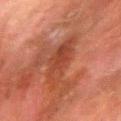| key | value |
|---|---|
| biopsy status | total-body-photography surveillance lesion; no biopsy |
| automated metrics | a footprint of about 13 mm², a shape eccentricity near 0.85, and a symmetry-axis asymmetry near 0.25; a border-irregularity index near 3.5/10 and a peripheral color-asymmetry measure near 1.5 |
| subject | male, approximately 80 years of age |
| anatomic site | the right forearm |
| lesion diameter | about 5.5 mm |
| lighting | cross-polarized illumination |
| image source | ~15 mm crop, total-body skin-cancer survey |